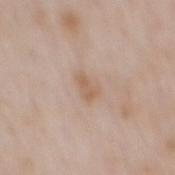workup=no biopsy performed (imaged during a skin exam)
site=the mid back
image=~15 mm crop, total-body skin-cancer survey
illumination=white-light
patient=male, aged around 65
lesion diameter=≈2.5 mm